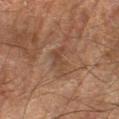Case summary:
* biopsy status: imaged on a skin check; not biopsied
* imaging modality: ~15 mm tile from a whole-body skin photo
* diameter: ≈3.5 mm
* subject: male, in their mid-60s
* location: the right forearm
* automated lesion analysis: a footprint of about 4.5 mm² and a shape eccentricity near 0.8
* tile lighting: cross-polarized illumination